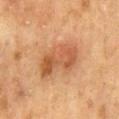{
  "biopsy_status": "not biopsied; imaged during a skin examination",
  "patient": {
    "sex": "male",
    "age_approx": 75
  },
  "site": "mid back",
  "image": {
    "source": "total-body photography crop",
    "field_of_view_mm": 15
  },
  "lighting": "cross-polarized"
}